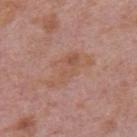The lesion was photographed on a routine skin check and not biopsied; there is no pathology result.